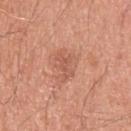Q: Is there a histopathology result?
A: catalogued during a skin exam; not biopsied
Q: How was this image acquired?
A: 15 mm crop, total-body photography
Q: Illumination type?
A: white-light
Q: What are the patient's age and sex?
A: male, aged around 50
Q: What is the anatomic site?
A: the back